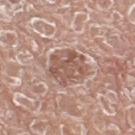The lesion was photographed on a routine skin check and not biopsied; there is no pathology result. Automated tile analysis of the lesion measured an area of roughly 13 mm², a shape eccentricity near 0.55, and two-axis asymmetry of about 0.2. It also reported a lesion color around L≈55 a*≈19 b*≈25 in CIELAB and a lesion-to-skin contrast of about 7 (normalized; higher = more distinct). It also reported an automated nevus-likeness rating near 0 out of 100 and a detector confidence of about 95 out of 100 that the crop contains a lesion. A male subject roughly 75 years of age. The tile uses white-light illumination. The recorded lesion diameter is about 4.5 mm. Located on the left lower leg. A 15 mm close-up extracted from a 3D total-body photography capture.